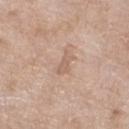image source: 15 mm crop, total-body photography
anatomic site: the left lower leg
patient: female, aged around 75